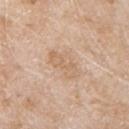Part of a total-body skin-imaging series; this lesion was reviewed on a skin check and was not flagged for biopsy.
From the chest.
A male patient, in their 80s.
The lesion's longest dimension is about 4.5 mm.
A close-up tile cropped from a whole-body skin photograph, about 15 mm across.
Automated image analysis of the tile measured a mean CIELAB color near L≈65 a*≈17 b*≈32, about 6 CIELAB-L* units darker than the surrounding skin, and a normalized lesion–skin contrast near 5. It also reported a nevus-likeness score of about 0/100 and a detector confidence of about 100 out of 100 that the crop contains a lesion.